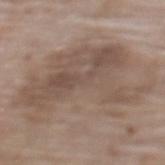workup: imaged on a skin check; not biopsied
lesion diameter: ~13 mm (longest diameter)
acquisition: 15 mm crop, total-body photography
site: the upper back
lighting: white-light
subject: female, aged approximately 75